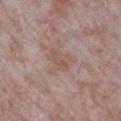Q: Is there a histopathology result?
A: total-body-photography surveillance lesion; no biopsy
Q: What lighting was used for the tile?
A: white-light
Q: Patient demographics?
A: male, aged around 65
Q: Automated lesion metrics?
A: a lesion area of about 5 mm², an eccentricity of roughly 0.75, and two-axis asymmetry of about 0.4; a classifier nevus-likeness of about 0/100 and a lesion-detection confidence of about 100/100
Q: Lesion location?
A: the arm
Q: What is the imaging modality?
A: ~15 mm crop, total-body skin-cancer survey
Q: Lesion size?
A: ≈3 mm